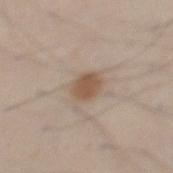| feature | finding |
|---|---|
| notes | total-body-photography surveillance lesion; no biopsy |
| location | the back |
| subject | male, about 35 years old |
| image | ~15 mm crop, total-body skin-cancer survey |
| diameter | about 2.5 mm |
| lighting | white-light |
| image-analysis metrics | an area of roughly 6 mm², an outline eccentricity of about 0.45 (0 = round, 1 = elongated), and two-axis asymmetry of about 0.15; an automated nevus-likeness rating near 100 out of 100 and a detector confidence of about 100 out of 100 that the crop contains a lesion |